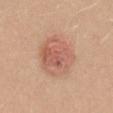Q: Is there a histopathology result?
A: imaged on a skin check; not biopsied
Q: Who is the patient?
A: female, approximately 20 years of age
Q: How was this image acquired?
A: total-body-photography crop, ~15 mm field of view
Q: Lesion location?
A: the mid back
Q: Automated lesion metrics?
A: a footprint of about 21 mm², an outline eccentricity of about 0.35 (0 = round, 1 = elongated), and a shape-asymmetry score of about 0.1 (0 = symmetric)
Q: What lighting was used for the tile?
A: white-light
Q: Lesion size?
A: ~5.5 mm (longest diameter)